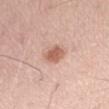<record>
<biopsy_status>not biopsied; imaged during a skin examination</biopsy_status>
<patient>
  <sex>male</sex>
  <age_approx>80</age_approx>
</patient>
<image>
  <source>total-body photography crop</source>
  <field_of_view_mm>15</field_of_view_mm>
</image>
<site>abdomen</site>
</record>